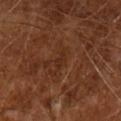biopsy status: imaged on a skin check; not biopsied
subject: male, approximately 65 years of age
lighting: cross-polarized illumination
image: ~15 mm tile from a whole-body skin photo